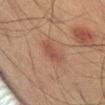biopsy status: catalogued during a skin exam; not biopsied
site: the back
lesion diameter: about 3 mm
image source: 15 mm crop, total-body photography
patient: male, aged 58 to 62
illumination: cross-polarized illumination